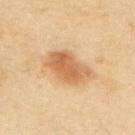{
  "biopsy_status": "not biopsied; imaged during a skin examination",
  "patient": {
    "sex": "female",
    "age_approx": 60
  },
  "lesion_size": {
    "long_diameter_mm_approx": 5.5
  },
  "automated_metrics": {
    "eccentricity": 0.85,
    "shape_asymmetry": 0.25,
    "nevus_likeness_0_100": 95,
    "lesion_detection_confidence_0_100": 100
  },
  "lighting": "cross-polarized",
  "image": {
    "source": "total-body photography crop",
    "field_of_view_mm": 15
  },
  "site": "upper back"
}